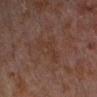The patient is a male aged 28–32.
The recorded lesion diameter is about 5.5 mm.
A roughly 15 mm field-of-view crop from a total-body skin photograph.
On the left forearm.